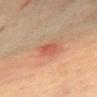<lesion>
  <patient>
    <sex>female</sex>
    <age_approx>65</age_approx>
  </patient>
  <automated_metrics>
    <area_mm2_approx>4.0</area_mm2_approx>
    <eccentricity>0.7</eccentricity>
    <shape_asymmetry>0.2</shape_asymmetry>
    <cielab_L>47</cielab_L>
    <cielab_a>26</cielab_a>
    <cielab_b>29</cielab_b>
    <vs_skin_darker_L>7.0</vs_skin_darker_L>
    <vs_skin_contrast_norm>5.5</vs_skin_contrast_norm>
    <nevus_likeness_0_100>0</nevus_likeness_0_100>
    <lesion_detection_confidence_0_100>100</lesion_detection_confidence_0_100>
  </automated_metrics>
  <lesion_size>
    <long_diameter_mm_approx>2.5</long_diameter_mm_approx>
  </lesion_size>
  <image>
    <source>total-body photography crop</source>
    <field_of_view_mm>15</field_of_view_mm>
  </image>
  <site>front of the torso</site>
  <lighting>cross-polarized</lighting>
</lesion>